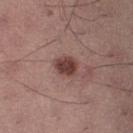biopsy status: total-body-photography surveillance lesion; no biopsy
imaging modality: total-body-photography crop, ~15 mm field of view
body site: the leg
tile lighting: white-light illumination
subject: male, about 40 years old
TBP lesion metrics: an area of roughly 6 mm², an outline eccentricity of about 0.55 (0 = round, 1 = elongated), and a symmetry-axis asymmetry near 0.2; a border-irregularity index near 2/10, a color-variation rating of about 3/10, and radial color variation of about 1; a nevus-likeness score of about 95/100 and a lesion-detection confidence of about 100/100
lesion diameter: ≈3 mm